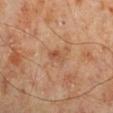An algorithmic analysis of the crop reported a shape eccentricity near 0.85. The analysis additionally found a lesion color around L≈50 a*≈23 b*≈33 in CIELAB and a lesion–skin lightness drop of about 8. It also reported internal color variation of about 3 on a 0–10 scale. The tile uses cross-polarized illumination. A male patient, aged around 70. The lesion is on the right lower leg. The recorded lesion diameter is about 2.5 mm. A region of skin cropped from a whole-body photographic capture, roughly 15 mm wide.